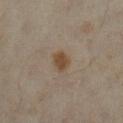follow-up: no biopsy performed (imaged during a skin exam) | image: 15 mm crop, total-body photography | subject: female, approximately 35 years of age | automated metrics: a border-irregularity index near 2.5/10 and peripheral color asymmetry of about 1; a nevus-likeness score of about 95/100 and lesion-presence confidence of about 100/100 | body site: the right lower leg | tile lighting: cross-polarized.